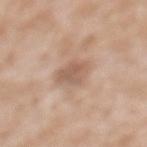<lesion>
  <biopsy_status>not biopsied; imaged during a skin examination</biopsy_status>
  <lesion_size>
    <long_diameter_mm_approx>3.5</long_diameter_mm_approx>
  </lesion_size>
  <automated_metrics>
    <area_mm2_approx>6.5</area_mm2_approx>
    <eccentricity>0.75</eccentricity>
    <shape_asymmetry>0.25</shape_asymmetry>
  </automated_metrics>
  <image>
    <source>total-body photography crop</source>
    <field_of_view_mm>15</field_of_view_mm>
  </image>
  <site>back</site>
  <patient>
    <sex>female</sex>
    <age_approx>40</age_approx>
  </patient>
  <lighting>white-light</lighting>
</lesion>